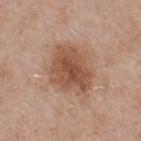lesion size = ~6 mm (longest diameter) | illumination = white-light | subject = male, aged around 55 | image = ~15 mm crop, total-body skin-cancer survey.